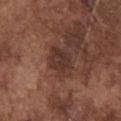Impression: No biopsy was performed on this lesion — it was imaged during a full skin examination and was not determined to be concerning. Background: This is a white-light tile. The lesion is located on the chest. The recorded lesion diameter is about 3.5 mm. A roughly 15 mm field-of-view crop from a total-body skin photograph. Automated image analysis of the tile measured a border-irregularity rating of about 2.5/10, a within-lesion color-variation index near 3/10, and peripheral color asymmetry of about 1. A male patient, in their mid- to late 70s.